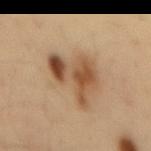This is a cross-polarized tile. The patient is a male in their mid-30s. Approximately 6 mm at its widest. Located on the mid back. A 15 mm crop from a total-body photograph taken for skin-cancer surveillance.